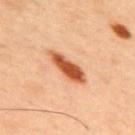Q: Was a biopsy performed?
A: no biopsy performed (imaged during a skin exam)
Q: Automated lesion metrics?
A: a lesion–skin lightness drop of about 17 and a normalized border contrast of about 11.5; border irregularity of about 3 on a 0–10 scale, a within-lesion color-variation index near 3.5/10, and radial color variation of about 1
Q: What kind of image is this?
A: 15 mm crop, total-body photography
Q: How large is the lesion?
A: ≈5.5 mm
Q: Who is the patient?
A: male, aged 43 to 47
Q: Illumination type?
A: cross-polarized illumination
Q: Lesion location?
A: the upper back